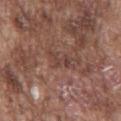Imaged during a routine full-body skin examination; the lesion was not biopsied and no histopathology is available.
A 15 mm crop from a total-body photograph taken for skin-cancer surveillance.
From the chest.
The patient is a male roughly 75 years of age.